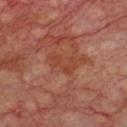{
  "biopsy_status": "not biopsied; imaged during a skin examination",
  "image": {
    "source": "total-body photography crop",
    "field_of_view_mm": 15
  },
  "site": "chest",
  "patient": {
    "sex": "male",
    "age_approx": 70
  }
}